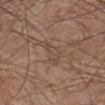| field | value |
|---|---|
| notes | no biopsy performed (imaged during a skin exam) |
| patient | male, aged approximately 60 |
| size | ~3.5 mm (longest diameter) |
| image source | ~15 mm tile from a whole-body skin photo |
| lighting | white-light |
| body site | the right lower leg |